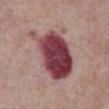Assessment: Part of a total-body skin-imaging series; this lesion was reviewed on a skin check and was not flagged for biopsy. Acquisition and patient details: An algorithmic analysis of the crop reported a mean CIELAB color near L≈42 a*≈28 b*≈17, about 20 CIELAB-L* units darker than the surrounding skin, and a normalized lesion–skin contrast near 14.5. The lesion is located on the front of the torso. The lesion's longest dimension is about 8 mm. A male subject aged 63 to 67. Cropped from a total-body skin-imaging series; the visible field is about 15 mm.